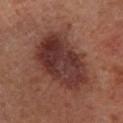Clinical impression: The lesion was photographed on a routine skin check and not biopsied; there is no pathology result. Clinical summary: A 15 mm close-up tile from a total-body photography series done for melanoma screening. The lesion is on the right lower leg. A male patient aged 63–67. Automated tile analysis of the lesion measured a border-irregularity rating of about 3/10, a color-variation rating of about 6/10, and radial color variation of about 2. Imaged with cross-polarized lighting. Longest diameter approximately 8 mm.